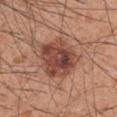Image and clinical context:
From the right upper arm. A male subject, approximately 60 years of age. A 15 mm close-up extracted from a 3D total-body photography capture.